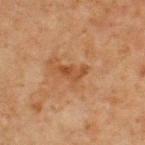{"biopsy_status": "not biopsied; imaged during a skin examination", "site": "chest", "patient": {"sex": "male", "age_approx": 65}, "image": {"source": "total-body photography crop", "field_of_view_mm": 15}}